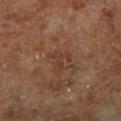Recorded during total-body skin imaging; not selected for excision or biopsy.
Approximately 3 mm at its widest.
A 15 mm close-up tile from a total-body photography series done for melanoma screening.
Automated tile analysis of the lesion measured a footprint of about 4 mm², an eccentricity of roughly 0.7, and two-axis asymmetry of about 0.6. And it measured a border-irregularity index near 8.5/10, internal color variation of about 0 on a 0–10 scale, and peripheral color asymmetry of about 0. The analysis additionally found an automated nevus-likeness rating near 0 out of 100 and a detector confidence of about 90 out of 100 that the crop contains a lesion.
Imaged with cross-polarized lighting.
A male patient, in their mid-60s.